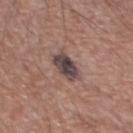workup — total-body-photography surveillance lesion; no biopsy
image — ~15 mm tile from a whole-body skin photo
location — the chest
lesion diameter — about 3.5 mm
lighting — white-light
subject — male, aged approximately 65